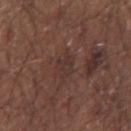Clinical impression: Part of a total-body skin-imaging series; this lesion was reviewed on a skin check and was not flagged for biopsy. Background: This is a white-light tile. Automated tile analysis of the lesion measured a mean CIELAB color near L≈33 a*≈17 b*≈20, a lesion–skin lightness drop of about 5, and a normalized lesion–skin contrast near 5. The software also gave a border-irregularity rating of about 2.5/10, a color-variation rating of about 2.5/10, and a peripheral color-asymmetry measure near 1. Cropped from a total-body skin-imaging series; the visible field is about 15 mm. On the left upper arm. The subject is a male aged 63–67. Measured at roughly 3 mm in maximum diameter.